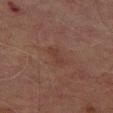Captured during whole-body skin photography for melanoma surveillance; the lesion was not biopsied.
The lesion-visualizer software estimated border irregularity of about 3 on a 0–10 scale, a color-variation rating of about 0/10, and radial color variation of about 0.
A male patient aged around 70.
The tile uses cross-polarized illumination.
The lesion is on the right thigh.
A region of skin cropped from a whole-body photographic capture, roughly 15 mm wide.